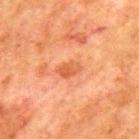Q: Was this lesion biopsied?
A: imaged on a skin check; not biopsied
Q: What is the imaging modality?
A: ~15 mm tile from a whole-body skin photo
Q: What did automated image analysis measure?
A: a lesion area of about 4 mm²; roughly 8 lightness units darker than nearby skin and a normalized border contrast of about 6.5
Q: What lighting was used for the tile?
A: cross-polarized
Q: Who is the patient?
A: male, aged 78 to 82
Q: Lesion size?
A: about 3 mm
Q: What is the anatomic site?
A: the mid back